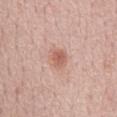Findings:
• workup · no biopsy performed (imaged during a skin exam)
• image source · 15 mm crop, total-body photography
• subject · male, aged around 60
• site · the chest
• size · ~2.5 mm (longest diameter)